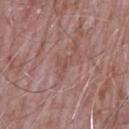Case summary:
• notes — imaged on a skin check; not biopsied
• lesion size — about 2.5 mm
• patient — male, aged 63–67
• body site — the left upper arm
• illumination — white-light
• image source — 15 mm crop, total-body photography
• automated metrics — an average lesion color of about L≈50 a*≈21 b*≈24 (CIELAB) and a lesion–skin lightness drop of about 6